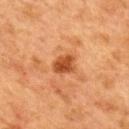Captured during whole-body skin photography for melanoma surveillance; the lesion was not biopsied. The patient is a female aged around 40. The lesion is on the mid back. A roughly 15 mm field-of-view crop from a total-body skin photograph. Measured at roughly 3 mm in maximum diameter. This is a cross-polarized tile.